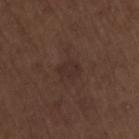subject: male, approximately 70 years of age; acquisition: ~15 mm tile from a whole-body skin photo; anatomic site: the lower back.